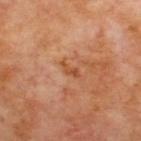image: total-body-photography crop, ~15 mm field of view | subject: male, aged approximately 70 | body site: the back | automated metrics: a lesion area of about 2.5 mm², a shape eccentricity near 0.9, and a shape-asymmetry score of about 0.45 (0 = symmetric); a lesion color around L≈50 a*≈27 b*≈38 in CIELAB and a lesion–skin lightness drop of about 8; a border-irregularity index near 5/10, internal color variation of about 0 on a 0–10 scale, and a peripheral color-asymmetry measure near 0; an automated nevus-likeness rating near 0 out of 100 and a lesion-detection confidence of about 100/100.